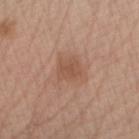Q: Was a biopsy performed?
A: catalogued during a skin exam; not biopsied
Q: How large is the lesion?
A: about 2.5 mm
Q: What is the imaging modality?
A: 15 mm crop, total-body photography
Q: What is the anatomic site?
A: the arm
Q: What are the patient's age and sex?
A: female, in their mid-60s